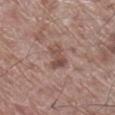Findings:
- notes: total-body-photography surveillance lesion; no biopsy
- tile lighting: white-light
- diameter: about 3.5 mm
- location: the right lower leg
- automated lesion analysis: an area of roughly 5 mm² and an eccentricity of roughly 0.85; a border-irregularity index near 4.5/10 and a within-lesion color-variation index near 3.5/10
- subject: male, aged 68–72
- imaging modality: ~15 mm tile from a whole-body skin photo